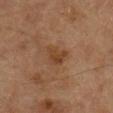| key | value |
|---|---|
| workup | imaged on a skin check; not biopsied |
| body site | the right lower leg |
| patient | male, roughly 85 years of age or older |
| size | ≈3 mm |
| lighting | cross-polarized illumination |
| imaging modality | total-body-photography crop, ~15 mm field of view |
| automated metrics | an outline eccentricity of about 0.65 (0 = round, 1 = elongated) and two-axis asymmetry of about 0.45; a border-irregularity index near 4.5/10, internal color variation of about 2 on a 0–10 scale, and radial color variation of about 0.5 |